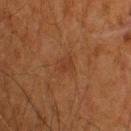Q: Is there a histopathology result?
A: no biopsy performed (imaged during a skin exam)
Q: What is the anatomic site?
A: the back
Q: Illumination type?
A: cross-polarized illumination
Q: Who is the patient?
A: male, in their mid- to late 60s
Q: What is the lesion's diameter?
A: ~2.5 mm (longest diameter)
Q: What kind of image is this?
A: 15 mm crop, total-body photography